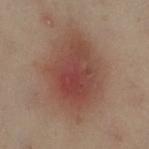<case>
  <biopsy_status>not biopsied; imaged during a skin examination</biopsy_status>
  <patient>
    <sex>female</sex>
    <age_approx>60</age_approx>
  </patient>
  <image>
    <source>total-body photography crop</source>
    <field_of_view_mm>15</field_of_view_mm>
  </image>
  <lighting>cross-polarized</lighting>
  <site>left leg</site>
</case>